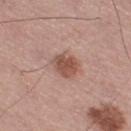{
  "biopsy_status": "not biopsied; imaged during a skin examination",
  "lesion_size": {
    "long_diameter_mm_approx": 3.0
  },
  "lighting": "white-light",
  "patient": {
    "sex": "male",
    "age_approx": 70
  },
  "site": "right thigh",
  "image": {
    "source": "total-body photography crop",
    "field_of_view_mm": 15
  },
  "automated_metrics": {
    "cielab_L": 52,
    "cielab_a": 22,
    "cielab_b": 26,
    "vs_skin_darker_L": 12.0,
    "vs_skin_contrast_norm": 8.5,
    "border_irregularity_0_10": 2.0,
    "color_variation_0_10": 3.0,
    "peripheral_color_asymmetry": 1.0
  }
}